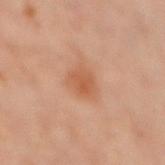No biopsy was performed on this lesion — it was imaged during a full skin examination and was not determined to be concerning.
A roughly 15 mm field-of-view crop from a total-body skin photograph.
The patient is a male roughly 60 years of age.
An algorithmic analysis of the crop reported a lesion area of about 7 mm², an eccentricity of roughly 0.65, and a shape-asymmetry score of about 0.3 (0 = symmetric). The analysis additionally found an average lesion color of about L≈53 a*≈23 b*≈33 (CIELAB), about 8 CIELAB-L* units darker than the surrounding skin, and a normalized border contrast of about 6.5. It also reported a border-irregularity index near 2.5/10, internal color variation of about 3 on a 0–10 scale, and a peripheral color-asymmetry measure near 1. The analysis additionally found a nevus-likeness score of about 60/100.
This is a cross-polarized tile.
Approximately 3.5 mm at its widest.
Located on the back.